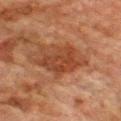Q: Was a biopsy performed?
A: total-body-photography surveillance lesion; no biopsy
Q: Who is the patient?
A: male, about 75 years old
Q: Where on the body is the lesion?
A: the chest
Q: What kind of image is this?
A: 15 mm crop, total-body photography
Q: Automated lesion metrics?
A: a lesion color around L≈36 a*≈23 b*≈30 in CIELAB, a lesion–skin lightness drop of about 9, and a lesion-to-skin contrast of about 8 (normalized; higher = more distinct); a border-irregularity index near 5.5/10, internal color variation of about 3 on a 0–10 scale, and radial color variation of about 1
Q: Illumination type?
A: cross-polarized
Q: What is the lesion's diameter?
A: ~6.5 mm (longest diameter)